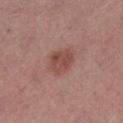From the left lower leg. About 3.5 mm across. The lesion-visualizer software estimated an area of roughly 8 mm², a shape eccentricity near 0.55, and a shape-asymmetry score of about 0.15 (0 = symmetric). It also reported a lesion color around L≈48 a*≈23 b*≈25 in CIELAB and roughly 8 lightness units darker than nearby skin. A female subject, aged 38–42. The tile uses white-light illumination. This image is a 15 mm lesion crop taken from a total-body photograph.